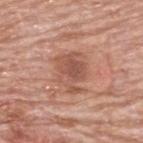| feature | finding |
|---|---|
| workup | catalogued during a skin exam; not biopsied |
| body site | the back |
| lesion diameter | about 4.5 mm |
| subject | male, roughly 80 years of age |
| image | ~15 mm tile from a whole-body skin photo |